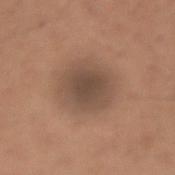patient: male, in their mid- to late 60s; image: 15 mm crop, total-body photography; location: the right forearm; lighting: white-light illumination.